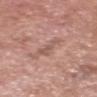{
  "biopsy_status": "not biopsied; imaged during a skin examination",
  "image": {
    "source": "total-body photography crop",
    "field_of_view_mm": 15
  },
  "patient": {
    "sex": "male",
    "age_approx": 60
  },
  "lighting": "white-light",
  "site": "head or neck"
}